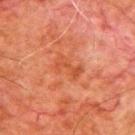Findings:
• notes — total-body-photography surveillance lesion; no biopsy